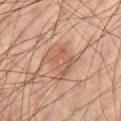The lesion was photographed on a routine skin check and not biopsied; there is no pathology result. Cropped from a total-body skin-imaging series; the visible field is about 15 mm. Located on the leg. Imaged with cross-polarized lighting. A male subject about 60 years old. Automated image analysis of the tile measured an eccentricity of roughly 0.5 and a symmetry-axis asymmetry near 0.3. The analysis additionally found an average lesion color of about L≈56 a*≈21 b*≈30 (CIELAB). About 4 mm across.